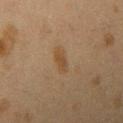| feature | finding |
|---|---|
| image-analysis metrics | lesion-presence confidence of about 100/100 |
| location | the right upper arm |
| imaging modality | total-body-photography crop, ~15 mm field of view |
| subject | female, approximately 40 years of age |
| size | ≈2.5 mm |